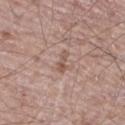follow-up=total-body-photography surveillance lesion; no biopsy
lighting=white-light illumination
subject=male, aged around 70
lesion diameter=about 2.5 mm
body site=the leg
image source=~15 mm tile from a whole-body skin photo